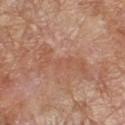follow-up: imaged on a skin check; not biopsied | tile lighting: cross-polarized | imaging modality: 15 mm crop, total-body photography | anatomic site: the right forearm | TBP lesion metrics: a lesion color around L≈54 a*≈22 b*≈31 in CIELAB and about 6 CIELAB-L* units darker than the surrounding skin; a color-variation rating of about 1.5/10 and a peripheral color-asymmetry measure near 0.5; a classifier nevus-likeness of about 0/100 and a lesion-detection confidence of about 80/100 | subject: female, aged around 55 | size: ≈7 mm.